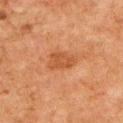Q: Is there a histopathology result?
A: imaged on a skin check; not biopsied
Q: Lesion location?
A: the upper back
Q: How was the tile lit?
A: cross-polarized
Q: What is the imaging modality?
A: ~15 mm tile from a whole-body skin photo
Q: What are the patient's age and sex?
A: male, about 80 years old